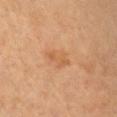Imaged during a routine full-body skin examination; the lesion was not biopsied and no histopathology is available. Located on the right arm. Automated image analysis of the tile measured a border-irregularity rating of about 5/10 and a within-lesion color-variation index near 1.5/10. The analysis additionally found an automated nevus-likeness rating near 0 out of 100 and lesion-presence confidence of about 100/100. Cropped from a whole-body photographic skin survey; the tile spans about 15 mm. The recorded lesion diameter is about 3.5 mm. This is a cross-polarized tile. A male patient aged around 60.